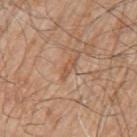| field | value |
|---|---|
| workup | no biopsy performed (imaged during a skin exam) |